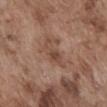<tbp_lesion>
  <biopsy_status>not biopsied; imaged during a skin examination</biopsy_status>
  <patient>
    <sex>male</sex>
    <age_approx>75</age_approx>
  </patient>
  <lesion_size>
    <long_diameter_mm_approx>4.5</long_diameter_mm_approx>
  </lesion_size>
  <image>
    <source>total-body photography crop</source>
    <field_of_view_mm>15</field_of_view_mm>
  </image>
  <lighting>white-light</lighting>
  <site>front of the torso</site>
</tbp_lesion>